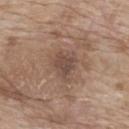The lesion is located on the upper back. The tile uses white-light illumination. The subject is a female approximately 75 years of age. The lesion's longest dimension is about 2.5 mm. Cropped from a whole-body photographic skin survey; the tile spans about 15 mm.